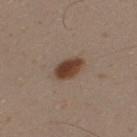<tbp_lesion>
<biopsy_status>not biopsied; imaged during a skin examination</biopsy_status>
<patient>
  <sex>male</sex>
  <age_approx>30</age_approx>
</patient>
<automated_metrics>
  <cielab_L>41</cielab_L>
  <cielab_a>18</cielab_a>
  <cielab_b>27</cielab_b>
  <vs_skin_darker_L>14.0</vs_skin_darker_L>
  <vs_skin_contrast_norm>11.5</vs_skin_contrast_norm>
  <nevus_likeness_0_100>100</nevus_likeness_0_100>
  <lesion_detection_confidence_0_100>100</lesion_detection_confidence_0_100>
</automated_metrics>
<image>
  <source>total-body photography crop</source>
  <field_of_view_mm>15</field_of_view_mm>
</image>
<site>upper back</site>
<lesion_size>
  <long_diameter_mm_approx>3.5</long_diameter_mm_approx>
</lesion_size>
<lighting>white-light</lighting>
</tbp_lesion>